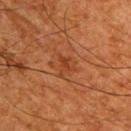Clinical impression: This lesion was catalogued during total-body skin photography and was not selected for biopsy. Context: The lesion is on the back. The total-body-photography lesion software estimated a lesion area of about 3.5 mm² and an outline eccentricity of about 0.7 (0 = round, 1 = elongated). The analysis additionally found a mean CIELAB color near L≈34 a*≈24 b*≈33 and roughly 6 lightness units darker than nearby skin. The software also gave border irregularity of about 5.5 on a 0–10 scale and a within-lesion color-variation index near 1.5/10. And it measured a classifier nevus-likeness of about 0/100. A male patient, in their mid-60s. A lesion tile, about 15 mm wide, cut from a 3D total-body photograph. The lesion's longest dimension is about 2.5 mm.